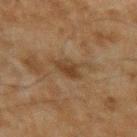The lesion was photographed on a routine skin check and not biopsied; there is no pathology result. Automated image analysis of the tile measured a footprint of about 4.5 mm². The analysis additionally found a lesion color around L≈33 a*≈15 b*≈27 in CIELAB and a lesion–skin lightness drop of about 7. It also reported border irregularity of about 2 on a 0–10 scale, internal color variation of about 2 on a 0–10 scale, and radial color variation of about 0.5. And it measured a classifier nevus-likeness of about 10/100 and a lesion-detection confidence of about 100/100. About 3 mm across. A roughly 15 mm field-of-view crop from a total-body skin photograph. The patient is a male about 45 years old. This is a cross-polarized tile. The lesion is located on the left upper arm.